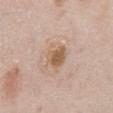Impression: Captured during whole-body skin photography for melanoma surveillance; the lesion was not biopsied. Context: Longest diameter approximately 3 mm. The lesion is located on the front of the torso. The total-body-photography lesion software estimated a within-lesion color-variation index near 3.5/10 and peripheral color asymmetry of about 1. The subject is a male in their 50s. A 15 mm close-up extracted from a 3D total-body photography capture.